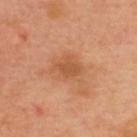Assessment: The lesion was tiled from a total-body skin photograph and was not biopsied. Image and clinical context: A roughly 15 mm field-of-view crop from a total-body skin photograph. Approximately 2.5 mm at its widest. Imaged with cross-polarized lighting. A female patient about 40 years old. From the upper back.